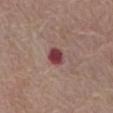The lesion was photographed on a routine skin check and not biopsied; there is no pathology result. A 15 mm crop from a total-body photograph taken for skin-cancer surveillance. The tile uses white-light illumination. The total-body-photography lesion software estimated a lesion area of about 5 mm² and an outline eccentricity of about 0.6 (0 = round, 1 = elongated). And it measured an average lesion color of about L≈42 a*≈27 b*≈19 (CIELAB). And it measured a border-irregularity index near 1.5/10, a color-variation rating of about 3/10, and a peripheral color-asymmetry measure near 1. The patient is a male aged 78 to 82. Located on the abdomen.